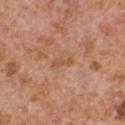Impression: This lesion was catalogued during total-body skin photography and was not selected for biopsy. Acquisition and patient details: A male patient aged around 65. On the chest. The lesion-visualizer software estimated a footprint of about 3 mm² and a shape eccentricity near 0.9. The software also gave a border-irregularity index near 4/10, a within-lesion color-variation index near 0/10, and peripheral color asymmetry of about 0. The analysis additionally found a classifier nevus-likeness of about 0/100 and lesion-presence confidence of about 100/100. Longest diameter approximately 3 mm. Imaged with white-light lighting. This image is a 15 mm lesion crop taken from a total-body photograph.